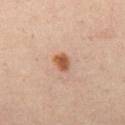Impression:
No biopsy was performed on this lesion — it was imaged during a full skin examination and was not determined to be concerning.
Acquisition and patient details:
A 15 mm close-up extracted from a 3D total-body photography capture. On the leg. Captured under cross-polarized illumination. A female subject aged 38 to 42. The lesion-visualizer software estimated a border-irregularity rating of about 1/10, internal color variation of about 3 on a 0–10 scale, and a peripheral color-asymmetry measure near 1. It also reported a nevus-likeness score of about 100/100 and lesion-presence confidence of about 100/100.